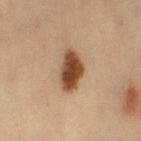Assessment:
The lesion was photographed on a routine skin check and not biopsied; there is no pathology result.
Acquisition and patient details:
A 15 mm close-up extracted from a 3D total-body photography capture. An algorithmic analysis of the crop reported an area of roughly 10 mm², an outline eccentricity of about 0.85 (0 = round, 1 = elongated), and a shape-asymmetry score of about 0.25 (0 = symmetric). It also reported a color-variation rating of about 4/10 and peripheral color asymmetry of about 1. It also reported a classifier nevus-likeness of about 100/100 and a detector confidence of about 100 out of 100 that the crop contains a lesion. The subject is a female aged 43 to 47. Located on the left thigh.